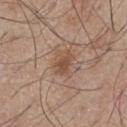• image · 15 mm crop, total-body photography
• lighting · white-light illumination
• location · the chest
• diameter · ~3 mm (longest diameter)
• patient · male, aged around 55